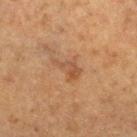The lesion was tiled from a total-body skin photograph and was not biopsied. An algorithmic analysis of the crop reported a lesion color around L≈41 a*≈18 b*≈29 in CIELAB, about 6 CIELAB-L* units darker than the surrounding skin, and a lesion-to-skin contrast of about 5.5 (normalized; higher = more distinct). It also reported internal color variation of about 3 on a 0–10 scale and peripheral color asymmetry of about 1. The software also gave an automated nevus-likeness rating near 0 out of 100 and lesion-presence confidence of about 100/100. A male subject roughly 75 years of age. Cropped from a whole-body photographic skin survey; the tile spans about 15 mm. Approximately 3.5 mm at its widest. From the right thigh. The tile uses cross-polarized illumination.